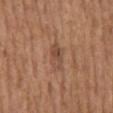* biopsy status · imaged on a skin check; not biopsied
* site · the mid back
* image · ~15 mm crop, total-body skin-cancer survey
* automated metrics · a footprint of about 6 mm² and a shape-asymmetry score of about 0.25 (0 = symmetric); a lesion color around L≈48 a*≈20 b*≈29 in CIELAB and a lesion–skin lightness drop of about 8; a border-irregularity rating of about 2.5/10, a color-variation rating of about 5/10, and peripheral color asymmetry of about 2
* illumination · white-light
* patient · female, aged approximately 65
* diameter · ≈3.5 mm